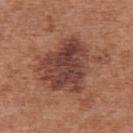Impression:
Imaged during a routine full-body skin examination; the lesion was not biopsied and no histopathology is available.
Clinical summary:
A roughly 15 mm field-of-view crop from a total-body skin photograph. Located on the upper back. Longest diameter approximately 7 mm. A female patient aged approximately 40. This is a white-light tile. An algorithmic analysis of the crop reported an area of roughly 29 mm² and a shape eccentricity near 0.55. The analysis additionally found an automated nevus-likeness rating near 10 out of 100 and lesion-presence confidence of about 100/100.